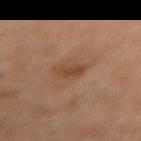Case summary:
* biopsy status — imaged on a skin check; not biopsied
* acquisition — total-body-photography crop, ~15 mm field of view
* size — ~3 mm (longest diameter)
* automated lesion analysis — an area of roughly 4 mm², an outline eccentricity of about 0.85 (0 = round, 1 = elongated), and a shape-asymmetry score of about 0.3 (0 = symmetric); a border-irregularity rating of about 3.5/10, a color-variation rating of about 1.5/10, and peripheral color asymmetry of about 0.5
* subject — female, aged 48 to 52
* location — the right thigh
* illumination — cross-polarized